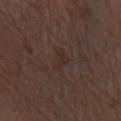Assessment:
Imaged during a routine full-body skin examination; the lesion was not biopsied and no histopathology is available.
Context:
The subject is a female aged 48–52. A close-up tile cropped from a whole-body skin photograph, about 15 mm across. On the left forearm.